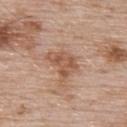- follow-up — no biopsy performed (imaged during a skin exam)
- lesion size — ~4 mm (longest diameter)
- patient — male, aged around 55
- anatomic site — the upper back
- tile lighting — white-light illumination
- image — total-body-photography crop, ~15 mm field of view
- TBP lesion metrics — an area of roughly 9 mm², an outline eccentricity of about 0.65 (0 = round, 1 = elongated), and a shape-asymmetry score of about 0.4 (0 = symmetric); a lesion color around L≈55 a*≈21 b*≈31 in CIELAB, about 9 CIELAB-L* units darker than the surrounding skin, and a normalized border contrast of about 6.5